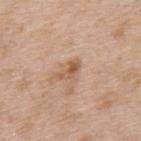Findings:
– follow-up: no biopsy performed (imaged during a skin exam)
– lesion diameter: about 3 mm
– subject: male, roughly 65 years of age
– site: the upper back
– image: ~15 mm crop, total-body skin-cancer survey